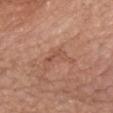The subject is a female aged approximately 75.
Cropped from a whole-body photographic skin survey; the tile spans about 15 mm.
On the mid back.